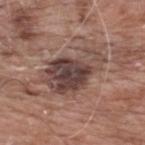<case>
  <biopsy_status>not biopsied; imaged during a skin examination</biopsy_status>
  <lighting>white-light</lighting>
  <automated_metrics>
    <border_irregularity_0_10>4.5</border_irregularity_0_10>
    <color_variation_0_10>7.5</color_variation_0_10>
    <peripheral_color_asymmetry>2.5</peripheral_color_asymmetry>
  </automated_metrics>
  <image>
    <source>total-body photography crop</source>
    <field_of_view_mm>15</field_of_view_mm>
  </image>
  <lesion_size>
    <long_diameter_mm_approx>7.0</long_diameter_mm_approx>
  </lesion_size>
  <patient>
    <sex>male</sex>
    <age_approx>60</age_approx>
  </patient>
  <site>back</site>
</case>